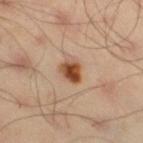No biopsy was performed on this lesion — it was imaged during a full skin examination and was not determined to be concerning. Imaged with cross-polarized lighting. Approximately 3 mm at its widest. A male patient aged approximately 45. Located on the right thigh. Cropped from a whole-body photographic skin survey; the tile spans about 15 mm.